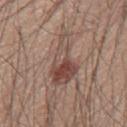| feature | finding |
|---|---|
| follow-up | catalogued during a skin exam; not biopsied |
| patient | male, about 60 years old |
| site | the mid back |
| acquisition | total-body-photography crop, ~15 mm field of view |
| size | about 7.5 mm |
| tile lighting | white-light |